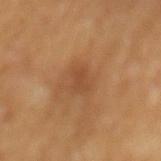Findings:
* follow-up — total-body-photography surveillance lesion; no biopsy
* lighting — cross-polarized illumination
* patient — male, aged around 70
* size — about 3 mm
* image — total-body-photography crop, ~15 mm field of view
* TBP lesion metrics — a lesion area of about 4.5 mm², a shape eccentricity near 0.8, and a shape-asymmetry score of about 0.25 (0 = symmetric)
* anatomic site — the mid back